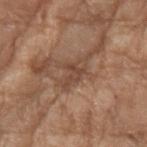notes: total-body-photography surveillance lesion; no biopsy | TBP lesion metrics: a mean CIELAB color near L≈45 a*≈19 b*≈28, roughly 8 lightness units darker than nearby skin, and a lesion-to-skin contrast of about 6 (normalized; higher = more distinct); a color-variation rating of about 2.5/10 and a peripheral color-asymmetry measure near 1; lesion-presence confidence of about 50/100 | patient: female, roughly 75 years of age | lighting: white-light | body site: the right forearm | size: about 4 mm | imaging modality: total-body-photography crop, ~15 mm field of view.